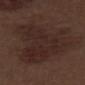Part of a total-body skin-imaging series; this lesion was reviewed on a skin check and was not flagged for biopsy.
The lesion is on the left thigh.
The patient is a male aged 68 to 72.
Imaged with white-light lighting.
Automated image analysis of the tile measured a footprint of about 50 mm² and a symmetry-axis asymmetry near 0.5. It also reported a nevus-likeness score of about 10/100 and a detector confidence of about 100 out of 100 that the crop contains a lesion.
A region of skin cropped from a whole-body photographic capture, roughly 15 mm wide.
The lesion's longest dimension is about 10.5 mm.